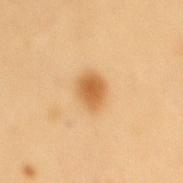Case summary:
• workup · imaged on a skin check; not biopsied
• automated metrics · a lesion area of about 7.5 mm² and an eccentricity of roughly 0.6; a mean CIELAB color near L≈60 a*≈21 b*≈43 and a lesion–skin lightness drop of about 12; a detector confidence of about 100 out of 100 that the crop contains a lesion
• diameter · about 3.5 mm
• imaging modality · ~15 mm crop, total-body skin-cancer survey
• subject · male, approximately 55 years of age
• anatomic site · the back
• tile lighting · cross-polarized